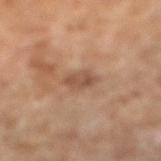<case>
<biopsy_status>not biopsied; imaged during a skin examination</biopsy_status>
<site>right lower leg</site>
<automated_metrics>
  <area_mm2_approx>4.0</area_mm2_approx>
  <eccentricity>0.75</eccentricity>
  <shape_asymmetry>0.25</shape_asymmetry>
  <cielab_L>48</cielab_L>
  <cielab_a>19</cielab_a>
  <cielab_b>30</cielab_b>
  <vs_skin_contrast_norm>6.5</vs_skin_contrast_norm>
  <border_irregularity_0_10>2.5</border_irregularity_0_10>
  <color_variation_0_10>3.0</color_variation_0_10>
  <lesion_detection_confidence_0_100>100</lesion_detection_confidence_0_100>
</automated_metrics>
<image>
  <source>total-body photography crop</source>
  <field_of_view_mm>15</field_of_view_mm>
</image>
<patient>
  <sex>female</sex>
  <age_approx>70</age_approx>
</patient>
<lighting>cross-polarized</lighting>
</case>